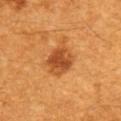Case summary:
– biopsy status — no biopsy performed (imaged during a skin exam)
– image source — ~15 mm crop, total-body skin-cancer survey
– diameter — ≈4 mm
– automated lesion analysis — a footprint of about 9.5 mm² and a symmetry-axis asymmetry near 0.2; a mean CIELAB color near L≈46 a*≈27 b*≈41, about 11 CIELAB-L* units darker than the surrounding skin, and a normalized border contrast of about 8.5; a border-irregularity rating of about 2/10, a within-lesion color-variation index near 4/10, and peripheral color asymmetry of about 1; an automated nevus-likeness rating near 90 out of 100
– lighting — cross-polarized illumination
– patient — male, about 60 years old
– body site — the upper back